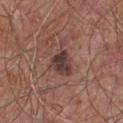workup = catalogued during a skin exam; not biopsied | site = the chest | image-analysis metrics = a lesion area of about 8 mm², a shape eccentricity near 0.8, and two-axis asymmetry of about 0.4; a color-variation rating of about 4.5/10 and a peripheral color-asymmetry measure near 1.5; an automated nevus-likeness rating near 60 out of 100 | lesion size = ≈5 mm | acquisition = ~15 mm crop, total-body skin-cancer survey | subject = male, aged around 65 | tile lighting = white-light illumination.